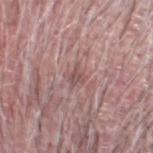notes = catalogued during a skin exam; not biopsied | lesion diameter = ≈2.5 mm | subject = male, roughly 70 years of age | body site = the head or neck | tile lighting = white-light illumination | imaging modality = ~15 mm crop, total-body skin-cancer survey.